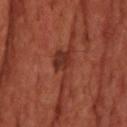follow-up = no biopsy performed (imaged during a skin exam); subject = male, aged approximately 65; imaging modality = ~15 mm tile from a whole-body skin photo; tile lighting = cross-polarized; location = the head or neck; diameter = about 6 mm.